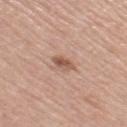automated lesion analysis: a footprint of about 3.5 mm² and an outline eccentricity of about 0.7 (0 = round, 1 = elongated); a lesion color around L≈55 a*≈21 b*≈28 in CIELAB and a normalized lesion–skin contrast near 7.5; a border-irregularity index near 2/10, a color-variation rating of about 3.5/10, and radial color variation of about 1.5
body site: the right thigh
acquisition: ~15 mm tile from a whole-body skin photo
subject: female, in their mid- to late 60s
lesion diameter: ≈2.5 mm